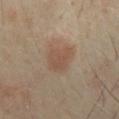Case summary:
– subject — male, aged around 65
– image-analysis metrics — an average lesion color of about L≈50 a*≈16 b*≈28 (CIELAB), roughly 7 lightness units darker than nearby skin, and a normalized lesion–skin contrast near 5.5; a classifier nevus-likeness of about 100/100 and lesion-presence confidence of about 100/100
– body site — the chest
– diameter — about 3.5 mm
– imaging modality — 15 mm crop, total-body photography
– tile lighting — cross-polarized illumination